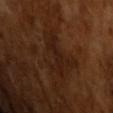No biopsy was performed on this lesion — it was imaged during a full skin examination and was not determined to be concerning. The subject is a male in their mid- to late 60s. A roughly 15 mm field-of-view crop from a total-body skin photograph. The recorded lesion diameter is about 4.5 mm. The tile uses cross-polarized illumination. Automated tile analysis of the lesion measured a lesion color around L≈18 a*≈18 b*≈22 in CIELAB, roughly 5 lightness units darker than nearby skin, and a normalized border contrast of about 7. The software also gave a border-irregularity rating of about 8/10 and internal color variation of about 1 on a 0–10 scale. The analysis additionally found a nevus-likeness score of about 0/100 and a detector confidence of about 80 out of 100 that the crop contains a lesion.